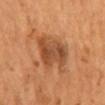{
  "biopsy_status": "not biopsied; imaged during a skin examination",
  "site": "mid back",
  "patient": {
    "sex": "male",
    "age_approx": 55
  },
  "lighting": "cross-polarized",
  "image": {
    "source": "total-body photography crop",
    "field_of_view_mm": 15
  }
}